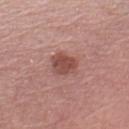Q: Was a biopsy performed?
A: imaged on a skin check; not biopsied
Q: How was this image acquired?
A: 15 mm crop, total-body photography
Q: What is the anatomic site?
A: the left upper arm
Q: What lighting was used for the tile?
A: white-light
Q: Who is the patient?
A: female, in their mid- to late 60s
Q: Lesion size?
A: ≈3.5 mm